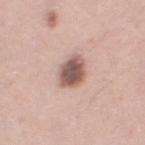The lesion was tiled from a total-body skin photograph and was not biopsied. The subject is a female aged approximately 40. About 3.5 mm across. This image is a 15 mm lesion crop taken from a total-body photograph. Imaged with white-light lighting. From the left thigh.